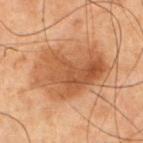Imaged during a routine full-body skin examination; the lesion was not biopsied and no histopathology is available. Captured under cross-polarized illumination. The total-body-photography lesion software estimated lesion-presence confidence of about 100/100. Located on the chest. Approximately 8.5 mm at its widest. A male patient, aged 68–72. A region of skin cropped from a whole-body photographic capture, roughly 15 mm wide.